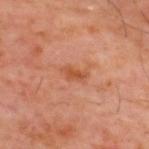Assessment:
The lesion was tiled from a total-body skin photograph and was not biopsied.
Image and clinical context:
A male patient, roughly 60 years of age. Imaged with cross-polarized lighting. A 15 mm crop from a total-body photograph taken for skin-cancer surveillance. Located on the upper back. Longest diameter approximately 3 mm.